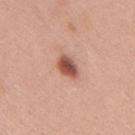<tbp_lesion>
<biopsy_status>not biopsied; imaged during a skin examination</biopsy_status>
<automated_metrics>
  <eccentricity>0.75</eccentricity>
  <shape_asymmetry>0.25</shape_asymmetry>
  <border_irregularity_0_10>2.5</border_irregularity_0_10>
  <peripheral_color_asymmetry>1.0</peripheral_color_asymmetry>
  <nevus_likeness_0_100>100</nevus_likeness_0_100>
  <lesion_detection_confidence_0_100>100</lesion_detection_confidence_0_100>
</automated_metrics>
<patient>
  <sex>female</sex>
  <age_approx>40</age_approx>
</patient>
<site>mid back</site>
<lesion_size>
  <long_diameter_mm_approx>3.0</long_diameter_mm_approx>
</lesion_size>
<lighting>white-light</lighting>
<image>
  <source>total-body photography crop</source>
  <field_of_view_mm>15</field_of_view_mm>
</image>
</tbp_lesion>